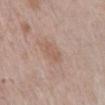Clinical impression: Part of a total-body skin-imaging series; this lesion was reviewed on a skin check and was not flagged for biopsy. Context: Cropped from a total-body skin-imaging series; the visible field is about 15 mm. Imaged with white-light lighting. The subject is a male aged 58 to 62. Approximately 2.5 mm at its widest. The lesion is located on the abdomen. An algorithmic analysis of the crop reported a lesion area of about 3 mm², a shape eccentricity near 0.85, and a symmetry-axis asymmetry near 0.4. And it measured an average lesion color of about L≈57 a*≈18 b*≈27 (CIELAB), about 6 CIELAB-L* units darker than the surrounding skin, and a normalized border contrast of about 5.